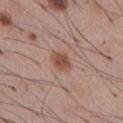Part of a total-body skin-imaging series; this lesion was reviewed on a skin check and was not flagged for biopsy.
A 15 mm crop from a total-body photograph taken for skin-cancer surveillance.
Located on the abdomen.
Captured under white-light illumination.
The subject is a male aged 53 to 57.
Longest diameter approximately 3 mm.